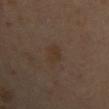Impression: Imaged during a routine full-body skin examination; the lesion was not biopsied and no histopathology is available. Context: This is a cross-polarized tile. The patient is a female roughly 40 years of age. The recorded lesion diameter is about 3 mm. The lesion is on the arm. Cropped from a whole-body photographic skin survey; the tile spans about 15 mm.